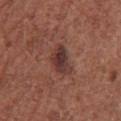Assessment:
This lesion was catalogued during total-body skin photography and was not selected for biopsy.
Clinical summary:
A 15 mm close-up tile from a total-body photography series done for melanoma screening. Located on the front of the torso. A male subject, aged approximately 75.